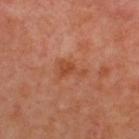{"biopsy_status": "not biopsied; imaged during a skin examination", "automated_metrics": {"border_irregularity_0_10": 6.5, "color_variation_0_10": 1.5, "peripheral_color_asymmetry": 0.5, "nevus_likeness_0_100": 0, "lesion_detection_confidence_0_100": 100}, "site": "upper back", "lesion_size": {"long_diameter_mm_approx": 3.5}, "lighting": "cross-polarized", "patient": {"sex": "male", "age_approx": 50}, "image": {"source": "total-body photography crop", "field_of_view_mm": 15}}